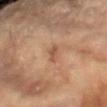Clinical impression:
This lesion was catalogued during total-body skin photography and was not selected for biopsy.
Acquisition and patient details:
Automated tile analysis of the lesion measured a lesion area of about 2 mm². It also reported a border-irregularity index near 4/10, internal color variation of about 0 on a 0–10 scale, and radial color variation of about 0. The software also gave a nevus-likeness score of about 0/100. Cropped from a total-body skin-imaging series; the visible field is about 15 mm. The lesion is on the right arm. A female patient, in their 80s. Longest diameter approximately 2.5 mm. The tile uses cross-polarized illumination.